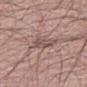Captured during whole-body skin photography for melanoma surveillance; the lesion was not biopsied.
Longest diameter approximately 3 mm.
A male patient, aged approximately 65.
Cropped from a whole-body photographic skin survey; the tile spans about 15 mm.
Captured under white-light illumination.
The lesion-visualizer software estimated an average lesion color of about L≈52 a*≈16 b*≈21 (CIELAB). The analysis additionally found a border-irregularity rating of about 2.5/10 and a color-variation rating of about 4/10.
The lesion is on the right thigh.